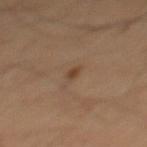{"site": "mid back", "image": {"source": "total-body photography crop", "field_of_view_mm": 15}, "lighting": "cross-polarized", "patient": {"sex": "male", "age_approx": 65}, "lesion_size": {"long_diameter_mm_approx": 1.5}, "automated_metrics": {"eccentricity": 0.7, "shape_asymmetry": 0.35, "cielab_L": 40, "cielab_a": 17, "cielab_b": 29, "vs_skin_contrast_norm": 7.0, "border_irregularity_0_10": 2.5, "color_variation_0_10": 1.5, "peripheral_color_asymmetry": 0.5}}